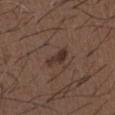Clinical summary: The lesion is on the front of the torso. This is a white-light tile. A 15 mm close-up tile from a total-body photography series done for melanoma screening. Longest diameter approximately 3.5 mm. A male subject roughly 50 years of age.